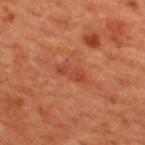{"biopsy_status": "not biopsied; imaged during a skin examination"}